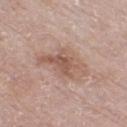The lesion was tiled from a total-body skin photograph and was not biopsied.
From the left thigh.
A region of skin cropped from a whole-body photographic capture, roughly 15 mm wide.
A male patient aged 58 to 62.